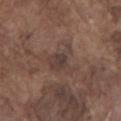Clinical impression: Captured during whole-body skin photography for melanoma surveillance; the lesion was not biopsied. Context: Imaged with white-light lighting. From the chest. A male subject, aged 73 to 77. The lesion's longest dimension is about 4.5 mm. Cropped from a whole-body photographic skin survey; the tile spans about 15 mm.